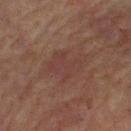Clinical impression: Recorded during total-body skin imaging; not selected for excision or biopsy. Context: Located on the left thigh. About 5 mm across. A female subject approximately 60 years of age. This image is a 15 mm lesion crop taken from a total-body photograph. The lesion-visualizer software estimated a footprint of about 12 mm², a shape eccentricity near 0.65, and a shape-asymmetry score of about 0.35 (0 = symmetric). The analysis additionally found a lesion color around L≈34 a*≈18 b*≈20 in CIELAB, roughly 4 lightness units darker than nearby skin, and a normalized lesion–skin contrast near 4.5. The analysis additionally found a border-irregularity index near 5/10 and radial color variation of about 0.5. The analysis additionally found a nevus-likeness score of about 0/100 and a lesion-detection confidence of about 100/100. Imaged with cross-polarized lighting.